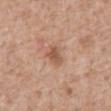follow-up: catalogued during a skin exam; not biopsied | illumination: white-light illumination | size: ~2.5 mm (longest diameter) | automated metrics: a shape eccentricity near 0.7; a mean CIELAB color near L≈55 a*≈21 b*≈32 and a normalized border contrast of about 7; border irregularity of about 2.5 on a 0–10 scale, a within-lesion color-variation index near 3/10, and a peripheral color-asymmetry measure near 1; an automated nevus-likeness rating near 40 out of 100 and a lesion-detection confidence of about 100/100 | location: the front of the torso | patient: male, aged around 60 | imaging modality: total-body-photography crop, ~15 mm field of view.